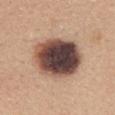Q: Is there a histopathology result?
A: total-body-photography surveillance lesion; no biopsy
Q: How large is the lesion?
A: ≈7 mm
Q: Illumination type?
A: white-light illumination
Q: How was this image acquired?
A: ~15 mm crop, total-body skin-cancer survey
Q: What did automated image analysis measure?
A: a border-irregularity index near 1/10, internal color variation of about 9.5 on a 0–10 scale, and peripheral color asymmetry of about 3
Q: Where on the body is the lesion?
A: the mid back
Q: What are the patient's age and sex?
A: female, roughly 35 years of age